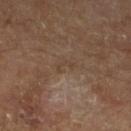No biopsy was performed on this lesion — it was imaged during a full skin examination and was not determined to be concerning. On the left lower leg. Cropped from a whole-body photographic skin survey; the tile spans about 15 mm. Automated image analysis of the tile measured a lesion area of about 2 mm², an eccentricity of roughly 0.95, and two-axis asymmetry of about 0.6. And it measured a mean CIELAB color near L≈40 a*≈14 b*≈26 and a lesion-to-skin contrast of about 4.5 (normalized; higher = more distinct). It also reported a border-irregularity rating of about 8/10, internal color variation of about 0 on a 0–10 scale, and a peripheral color-asymmetry measure near 0. It also reported a classifier nevus-likeness of about 0/100 and a detector confidence of about 60 out of 100 that the crop contains a lesion. The subject is in their mid-50s. The tile uses cross-polarized illumination.